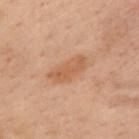No biopsy was performed on this lesion — it was imaged during a full skin examination and was not determined to be concerning. Imaged with cross-polarized lighting. On the mid back. About 4.5 mm across. A female patient, aged approximately 55. A roughly 15 mm field-of-view crop from a total-body skin photograph.